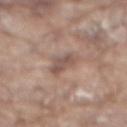biopsy status = total-body-photography surveillance lesion; no biopsy | diameter = about 3.5 mm | site = the mid back | imaging modality = 15 mm crop, total-body photography | TBP lesion metrics = a lesion area of about 5.5 mm², a shape eccentricity near 0.7, and a symmetry-axis asymmetry near 0.35; border irregularity of about 3.5 on a 0–10 scale, a within-lesion color-variation index near 2.5/10, and a peripheral color-asymmetry measure near 0.5; a classifier nevus-likeness of about 0/100 and a lesion-detection confidence of about 95/100 | patient = male, in their 80s | tile lighting = white-light illumination.